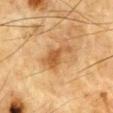Assessment:
Recorded during total-body skin imaging; not selected for excision or biopsy.
Acquisition and patient details:
Located on the chest. The tile uses cross-polarized illumination. Automated tile analysis of the lesion measured a lesion-to-skin contrast of about 7.5 (normalized; higher = more distinct). And it measured a nevus-likeness score of about 40/100. Cropped from a total-body skin-imaging series; the visible field is about 15 mm. A male patient roughly 85 years of age or older.